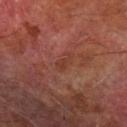Q: Was this lesion biopsied?
A: no biopsy performed (imaged during a skin exam)
Q: What are the patient's age and sex?
A: male, aged around 75
Q: What is the lesion's diameter?
A: ≈3 mm
Q: What is the anatomic site?
A: the left forearm
Q: How was this image acquired?
A: total-body-photography crop, ~15 mm field of view
Q: Automated lesion metrics?
A: a lesion color around L≈31 a*≈21 b*≈24 in CIELAB, a lesion–skin lightness drop of about 5, and a lesion-to-skin contrast of about 5.5 (normalized; higher = more distinct); a border-irregularity index near 3.5/10
Q: How was the tile lit?
A: cross-polarized illumination